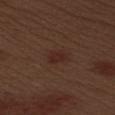notes — imaged on a skin check; not biopsied
tile lighting — white-light illumination
TBP lesion metrics — about 5 CIELAB-L* units darker than the surrounding skin
site — the left upper arm
lesion size — ≈2.5 mm
image — total-body-photography crop, ~15 mm field of view
subject — male, aged around 70